A male patient aged approximately 65, from the upper back, cropped from a whole-body photographic skin survey; the tile spans about 15 mm.
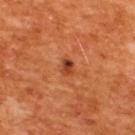  lesion_size:
    long_diameter_mm_approx: 2.0
  lighting: cross-polarized
  diagnosis:
    histopathology: seborrheic keratosis
    malignancy: benign
    taxonomic_path:
      - Benign
      - Benign epidermal proliferations
      - Seborrheic keratosis